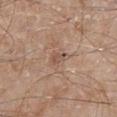Part of a total-body skin-imaging series; this lesion was reviewed on a skin check and was not flagged for biopsy.
A male patient, aged 63–67.
A 15 mm close-up extracted from a 3D total-body photography capture.
The lesion is on the right lower leg.